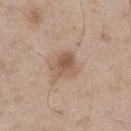  biopsy_status: not biopsied; imaged during a skin examination
  image:
    source: total-body photography crop
    field_of_view_mm: 15
  site: right thigh
  patient:
    sex: male
    age_approx: 60
  lesion_size:
    long_diameter_mm_approx: 3.0
  automated_metrics:
    vs_skin_darker_L: 10.0
    border_irregularity_0_10: 3.0
    color_variation_0_10: 4.0
    peripheral_color_asymmetry: 1.5
  lighting: white-light